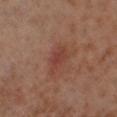– workup — catalogued during a skin exam; not biopsied
– anatomic site — the leg
– subject — male, aged 68 to 72
– acquisition — ~15 mm tile from a whole-body skin photo
– lesion diameter — ≈3.5 mm